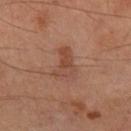biopsy status: total-body-photography surveillance lesion; no biopsy
size: ~4 mm (longest diameter)
site: the right thigh
image source: ~15 mm crop, total-body skin-cancer survey
patient: male, aged 63 to 67